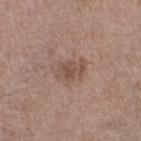The lesion was tiled from a total-body skin photograph and was not biopsied.
The lesion is located on the leg.
A male subject, aged 48–52.
A roughly 15 mm field-of-view crop from a total-body skin photograph.